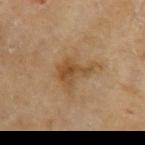Part of a total-body skin-imaging series; this lesion was reviewed on a skin check and was not flagged for biopsy. This is a cross-polarized tile. A close-up tile cropped from a whole-body skin photograph, about 15 mm across. The lesion is located on the left upper arm. A male patient aged approximately 70.